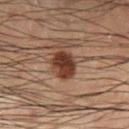biopsy status=total-body-photography surveillance lesion; no biopsy
acquisition=~15 mm crop, total-body skin-cancer survey
lesion diameter=~3.5 mm (longest diameter)
body site=the left lower leg
subject=male, about 50 years old
lighting=cross-polarized illumination